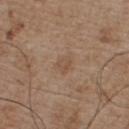workup: imaged on a skin check; not biopsied | size: about 2.5 mm | image-analysis metrics: a footprint of about 4.5 mm², an eccentricity of roughly 0.6, and a symmetry-axis asymmetry near 0.25; a border-irregularity index near 2.5/10, a color-variation rating of about 2/10, and radial color variation of about 0.5; an automated nevus-likeness rating near 0 out of 100 and a detector confidence of about 100 out of 100 that the crop contains a lesion | image source: 15 mm crop, total-body photography | lighting: white-light | subject: male, approximately 55 years of age | anatomic site: the chest.